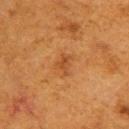| field | value |
|---|---|
| biopsy status | no biopsy performed (imaged during a skin exam) |
| image source | ~15 mm tile from a whole-body skin photo |
| location | the upper back |
| subject | female, approximately 40 years of age |
| lighting | cross-polarized |